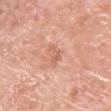Q: Was a biopsy performed?
A: catalogued during a skin exam; not biopsied
Q: What did automated image analysis measure?
A: border irregularity of about 6 on a 0–10 scale, a within-lesion color-variation index near 0.5/10, and radial color variation of about 0; a classifier nevus-likeness of about 0/100 and a lesion-detection confidence of about 100/100
Q: What is the imaging modality?
A: ~15 mm tile from a whole-body skin photo
Q: What lighting was used for the tile?
A: white-light illumination
Q: Who is the patient?
A: male, approximately 80 years of age
Q: Lesion location?
A: the chest
Q: Lesion size?
A: about 2.5 mm